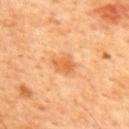{"biopsy_status": "not biopsied; imaged during a skin examination", "lighting": "cross-polarized", "site": "upper back", "image": {"source": "total-body photography crop", "field_of_view_mm": 15}, "patient": {"sex": "male", "age_approx": 45}, "automated_metrics": {"area_mm2_approx": 5.0, "eccentricity": 0.6, "shape_asymmetry": 0.3}, "lesion_size": {"long_diameter_mm_approx": 3.0}}